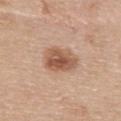This lesion was catalogued during total-body skin photography and was not selected for biopsy.
The lesion is located on the upper back.
The lesion's longest dimension is about 4 mm.
The tile uses white-light illumination.
A female patient in their mid- to late 60s.
A region of skin cropped from a whole-body photographic capture, roughly 15 mm wide.
Automated tile analysis of the lesion measured a lesion area of about 11 mm², an outline eccentricity of about 0.65 (0 = round, 1 = elongated), and a symmetry-axis asymmetry near 0.15. And it measured a border-irregularity rating of about 1.5/10 and radial color variation of about 1.5. The software also gave an automated nevus-likeness rating near 85 out of 100 and a detector confidence of about 100 out of 100 that the crop contains a lesion.